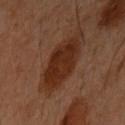Q: What lighting was used for the tile?
A: cross-polarized illumination
Q: Lesion location?
A: the right arm
Q: Who is the patient?
A: female, roughly 60 years of age
Q: What is the lesion's diameter?
A: ≈6.5 mm
Q: What is the imaging modality?
A: ~15 mm tile from a whole-body skin photo
Q: What did automated image analysis measure?
A: an area of roughly 19 mm², a shape eccentricity near 0.8, and two-axis asymmetry of about 0.15; border irregularity of about 2.5 on a 0–10 scale, a within-lesion color-variation index near 3/10, and a peripheral color-asymmetry measure near 1; an automated nevus-likeness rating near 100 out of 100 and lesion-presence confidence of about 100/100